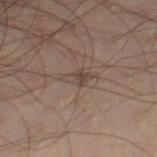Part of a total-body skin-imaging series; this lesion was reviewed on a skin check and was not flagged for biopsy.
From the left leg.
A male subject aged around 50.
A close-up tile cropped from a whole-body skin photograph, about 15 mm across.